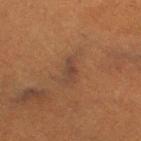image source — 15 mm crop, total-body photography
automated metrics — a mean CIELAB color near L≈34 a*≈17 b*≈24 and about 6 CIELAB-L* units darker than the surrounding skin; a border-irregularity rating of about 3.5/10 and radial color variation of about 0
lesion diameter — ~2.5 mm (longest diameter)
subject — female, aged approximately 55
body site — the right thigh
tile lighting — cross-polarized illumination